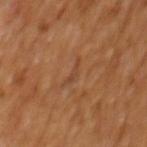Part of a total-body skin-imaging series; this lesion was reviewed on a skin check and was not flagged for biopsy. Automated image analysis of the tile measured a mean CIELAB color near L≈41 a*≈20 b*≈32, roughly 5 lightness units darker than nearby skin, and a lesion-to-skin contrast of about 4.5 (normalized; higher = more distinct). A male patient, aged 63 to 67. From the mid back. A 15 mm crop from a total-body photograph taken for skin-cancer surveillance.